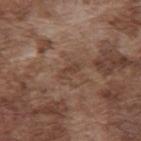Imaged during a routine full-body skin examination; the lesion was not biopsied and no histopathology is available.
Measured at roughly 2.5 mm in maximum diameter.
Automated image analysis of the tile measured an area of roughly 2 mm² and a shape-asymmetry score of about 0.45 (0 = symmetric). The analysis additionally found a color-variation rating of about 0/10.
Imaged with white-light lighting.
A male patient roughly 75 years of age.
A 15 mm crop from a total-body photograph taken for skin-cancer surveillance.
From the mid back.